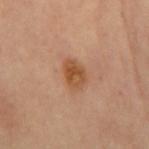<record>
  <biopsy_status>not biopsied; imaged during a skin examination</biopsy_status>
  <lesion_size>
    <long_diameter_mm_approx>3.5</long_diameter_mm_approx>
  </lesion_size>
  <automated_metrics>
    <border_irregularity_0_10>2.0</border_irregularity_0_10>
    <color_variation_0_10>5.0</color_variation_0_10>
    <nevus_likeness_0_100>85</nevus_likeness_0_100>
  </automated_metrics>
  <site>mid back</site>
  <lighting>cross-polarized</lighting>
  <image>
    <source>total-body photography crop</source>
    <field_of_view_mm>15</field_of_view_mm>
  </image>
</record>